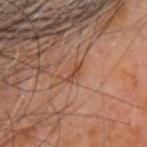{"biopsy_status": "not biopsied; imaged during a skin examination"}